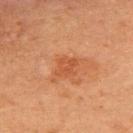Background:
A female patient roughly 50 years of age. Located on the back. Approximately 3 mm at its widest. A roughly 15 mm field-of-view crop from a total-body skin photograph. The tile uses cross-polarized illumination.